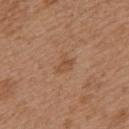workup: catalogued during a skin exam; not biopsied | patient: female, approximately 40 years of age | anatomic site: the left upper arm | acquisition: total-body-photography crop, ~15 mm field of view.